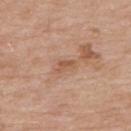notes: total-body-photography surveillance lesion; no biopsy | diameter: ≈2.5 mm | illumination: white-light | imaging modality: total-body-photography crop, ~15 mm field of view | body site: the upper back | subject: male, aged around 75.